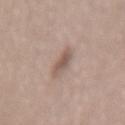The lesion was photographed on a routine skin check and not biopsied; there is no pathology result.
Located on the back.
A female subject, about 55 years old.
A close-up tile cropped from a whole-body skin photograph, about 15 mm across.